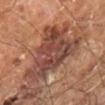Impression: Imaged during a routine full-body skin examination; the lesion was not biopsied and no histopathology is available. Background: A lesion tile, about 15 mm wide, cut from a 3D total-body photograph. Located on the leg. A male patient, aged approximately 60.